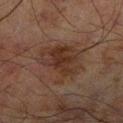{
  "site": "right lower leg",
  "image": {
    "source": "total-body photography crop",
    "field_of_view_mm": 15
  },
  "patient": {
    "sex": "male",
    "age_approx": 70
  }
}